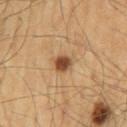Q: What is the anatomic site?
A: the mid back
Q: Patient demographics?
A: male, aged 58–62
Q: Illumination type?
A: cross-polarized illumination
Q: How was this image acquired?
A: ~15 mm crop, total-body skin-cancer survey
Q: What did automated image analysis measure?
A: a footprint of about 4 mm² and a shape eccentricity near 0.4; a lesion–skin lightness drop of about 15 and a lesion-to-skin contrast of about 10.5 (normalized; higher = more distinct)